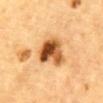Clinical impression:
Captured during whole-body skin photography for melanoma surveillance; the lesion was not biopsied.
Clinical summary:
Captured under cross-polarized illumination. Cropped from a whole-body photographic skin survey; the tile spans about 15 mm. A male patient, aged around 85 or older. The lesion is on the abdomen.